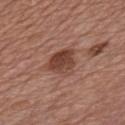<case>
<biopsy_status>not biopsied; imaged during a skin examination</biopsy_status>
<lighting>white-light</lighting>
<image>
  <source>total-body photography crop</source>
  <field_of_view_mm>15</field_of_view_mm>
</image>
<patient>
  <sex>male</sex>
  <age_approx>70</age_approx>
</patient>
<site>chest</site>
<lesion_size>
  <long_diameter_mm_approx>4.0</long_diameter_mm_approx>
</lesion_size>
<automated_metrics>
  <area_mm2_approx>9.5</area_mm2_approx>
  <eccentricity>0.55</eccentricity>
  <shape_asymmetry>0.3</shape_asymmetry>
  <nevus_likeness_0_100>90</nevus_likeness_0_100>
  <lesion_detection_confidence_0_100>100</lesion_detection_confidence_0_100>
</automated_metrics>
</case>